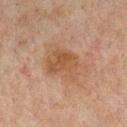Imaged during a routine full-body skin examination; the lesion was not biopsied and no histopathology is available. From the chest. A roughly 15 mm field-of-view crop from a total-body skin photograph. The lesion-visualizer software estimated a lesion color around L≈39 a*≈16 b*≈26 in CIELAB, a lesion–skin lightness drop of about 6, and a normalized lesion–skin contrast near 6.5. And it measured border irregularity of about 3 on a 0–10 scale, a within-lesion color-variation index near 3.5/10, and a peripheral color-asymmetry measure near 1. The patient is a male roughly 60 years of age.